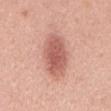<tbp_lesion>
  <biopsy_status>not biopsied; imaged during a skin examination</biopsy_status>
  <patient>
    <sex>male</sex>
    <age_approx>55</age_approx>
  </patient>
  <site>mid back</site>
  <image>
    <source>total-body photography crop</source>
    <field_of_view_mm>15</field_of_view_mm>
  </image>
  <lighting>white-light</lighting>
</tbp_lesion>